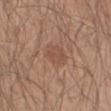Assessment: The lesion was tiled from a total-body skin photograph and was not biopsied. Acquisition and patient details: A close-up tile cropped from a whole-body skin photograph, about 15 mm across. The lesion is on the arm. Automated tile analysis of the lesion measured an area of roughly 7.5 mm², an outline eccentricity of about 0.55 (0 = round, 1 = elongated), and two-axis asymmetry of about 0.2. A male subject in their mid-30s. Measured at roughly 3.5 mm in maximum diameter. The tile uses white-light illumination.